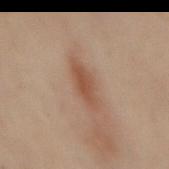Imaged during a routine full-body skin examination; the lesion was not biopsied and no histopathology is available.
The lesion is located on the mid back.
The lesion's longest dimension is about 5 mm.
A close-up tile cropped from a whole-body skin photograph, about 15 mm across.
The patient is a male aged around 50.
The tile uses cross-polarized illumination.